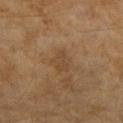| key | value |
|---|---|
| diameter | ~2.5 mm (longest diameter) |
| patient | female, aged 58 to 62 |
| location | the right forearm |
| acquisition | 15 mm crop, total-body photography |
| illumination | cross-polarized |